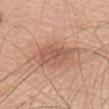Clinical impression: Imaged during a routine full-body skin examination; the lesion was not biopsied and no histopathology is available. Context: Imaged with white-light lighting. From the head or neck. A female subject, aged 38 to 42. About 4.5 mm across. This image is a 15 mm lesion crop taken from a total-body photograph.